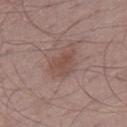Q: Is there a histopathology result?
A: imaged on a skin check; not biopsied
Q: Who is the patient?
A: male, aged around 50
Q: What is the imaging modality?
A: ~15 mm tile from a whole-body skin photo
Q: Lesion location?
A: the right thigh
Q: How was the tile lit?
A: white-light